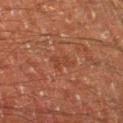Impression:
Part of a total-body skin-imaging series; this lesion was reviewed on a skin check and was not flagged for biopsy.
Clinical summary:
Captured under cross-polarized illumination. Approximately 3 mm at its widest. The lesion is located on the left thigh. A male subject, aged 58–62. Cropped from a total-body skin-imaging series; the visible field is about 15 mm.